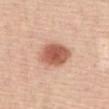workup: no biopsy performed (imaged during a skin exam) | imaging modality: ~15 mm crop, total-body skin-cancer survey | anatomic site: the chest | subject: female, approximately 55 years of age.